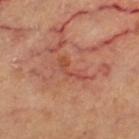Clinical impression: Recorded during total-body skin imaging; not selected for excision or biopsy. Clinical summary: Measured at roughly 4 mm in maximum diameter. This is a cross-polarized tile. A female subject in their 60s. The total-body-photography lesion software estimated a footprint of about 3.5 mm², an eccentricity of roughly 0.95, and a symmetry-axis asymmetry near 0.6. The software also gave a mean CIELAB color near L≈50 a*≈30 b*≈33 and roughly 7 lightness units darker than nearby skin. And it measured a border-irregularity index near 8.5/10, a color-variation rating of about 0/10, and radial color variation of about 0. Cropped from a whole-body photographic skin survey; the tile spans about 15 mm. From the left thigh.